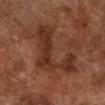Imaged during a routine full-body skin examination; the lesion was not biopsied and no histopathology is available.
A 15 mm close-up tile from a total-body photography series done for melanoma screening.
From the right lower leg.
A male subject in their 70s.
Automated image analysis of the tile measured an average lesion color of about L≈25 a*≈19 b*≈22 (CIELAB), about 6 CIELAB-L* units darker than the surrounding skin, and a normalized border contrast of about 7. The software also gave radial color variation of about 1. It also reported a nevus-likeness score of about 0/100 and a lesion-detection confidence of about 100/100.
Captured under cross-polarized illumination.